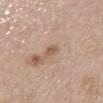notes: no biopsy performed (imaged during a skin exam)
body site: the mid back
lesion size: about 2.5 mm
automated lesion analysis: a border-irregularity index near 3.5/10, a color-variation rating of about 1.5/10, and peripheral color asymmetry of about 0.5
image: 15 mm crop, total-body photography
patient: female, about 65 years old